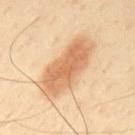Imaged during a routine full-body skin examination; the lesion was not biopsied and no histopathology is available. A male patient, aged 63 to 67. Located on the mid back. Captured under cross-polarized illumination. Cropped from a total-body skin-imaging series; the visible field is about 15 mm.